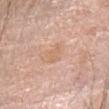Captured under white-light illumination.
A 15 mm close-up tile from a total-body photography series done for melanoma screening.
The lesion-visualizer software estimated an area of roughly 2.5 mm². The software also gave a peripheral color-asymmetry measure near 0. It also reported a classifier nevus-likeness of about 0/100 and lesion-presence confidence of about 100/100.
From the head or neck.
A female subject, aged 58–62.
The lesion's longest dimension is about 2.5 mm.